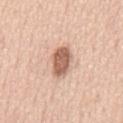The lesion was tiled from a total-body skin photograph and was not biopsied.
This image is a 15 mm lesion crop taken from a total-body photograph.
A male subject, in their mid- to late 60s.
From the front of the torso.
Longest diameter approximately 4.5 mm.